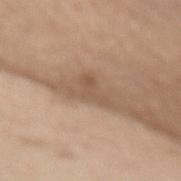Q: Is there a histopathology result?
A: imaged on a skin check; not biopsied
Q: Lesion size?
A: ≈4 mm
Q: What did automated image analysis measure?
A: roughly 8 lightness units darker than nearby skin and a normalized lesion–skin contrast near 6; a border-irregularity index near 8/10 and a within-lesion color-variation index near 1.5/10
Q: Who is the patient?
A: male, aged around 70
Q: What kind of image is this?
A: 15 mm crop, total-body photography
Q: How was the tile lit?
A: white-light
Q: What is the anatomic site?
A: the back